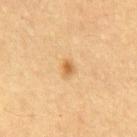follow-up: imaged on a skin check; not biopsied
automated lesion analysis: a lesion area of about 3 mm² and an eccentricity of roughly 0.7; a lesion color around L≈58 a*≈19 b*≈41 in CIELAB, a lesion–skin lightness drop of about 10, and a lesion-to-skin contrast of about 7 (normalized; higher = more distinct)
illumination: cross-polarized
lesion size: ≈2 mm
patient: male, aged approximately 60
body site: the chest
imaging modality: total-body-photography crop, ~15 mm field of view